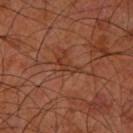{
  "biopsy_status": "not biopsied; imaged during a skin examination",
  "site": "left forearm",
  "patient": {
    "sex": "male",
    "age_approx": 60
  },
  "lighting": "cross-polarized",
  "lesion_size": {
    "long_diameter_mm_approx": 2.5
  },
  "image": {
    "source": "total-body photography crop",
    "field_of_view_mm": 15
  }
}